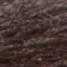Captured during whole-body skin photography for melanoma surveillance; the lesion was not biopsied. A 15 mm crop from a total-body photograph taken for skin-cancer surveillance. The lesion is on the abdomen. The subject is a male aged approximately 30.